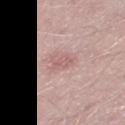No biopsy was performed on this lesion — it was imaged during a full skin examination and was not determined to be concerning. A lesion tile, about 15 mm wide, cut from a 3D total-body photograph. On the right thigh. The patient is a male in their 50s. Measured at roughly 2.5 mm in maximum diameter.